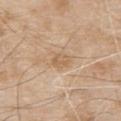Notes:
* biopsy status · no biopsy performed (imaged during a skin exam)
* patient · male, aged approximately 80
* TBP lesion metrics · a shape eccentricity near 0.7 and a symmetry-axis asymmetry near 0.2; a lesion color around L≈61 a*≈16 b*≈35 in CIELAB and a normalized border contrast of about 5.5; border irregularity of about 2 on a 0–10 scale, a within-lesion color-variation index near 3/10, and radial color variation of about 1; an automated nevus-likeness rating near 0 out of 100
* image source · 15 mm crop, total-body photography
* body site · the upper back